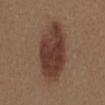Case summary:
– notes · catalogued during a skin exam; not biopsied
– size · about 8.5 mm
– acquisition · 15 mm crop, total-body photography
– automated metrics · a lesion color around L≈38 a*≈18 b*≈25 in CIELAB, about 12 CIELAB-L* units darker than the surrounding skin, and a normalized border contrast of about 10
– lighting · white-light illumination
– body site · the abdomen
– patient · female, aged 38 to 42